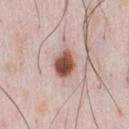{
  "biopsy_status": "not biopsied; imaged during a skin examination",
  "patient": {
    "sex": "male",
    "age_approx": 35
  },
  "site": "chest",
  "automated_metrics": {
    "area_mm2_approx": 7.0,
    "eccentricity": 0.7,
    "shape_asymmetry": 0.2,
    "cielab_L": 51,
    "cielab_a": 22,
    "cielab_b": 26,
    "vs_skin_contrast_norm": 13.0,
    "border_irregularity_0_10": 2.0,
    "color_variation_0_10": 5.5,
    "peripheral_color_asymmetry": 2.0
  },
  "image": {
    "source": "total-body photography crop",
    "field_of_view_mm": 15
  },
  "lighting": "white-light"
}